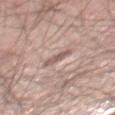The lesion was photographed on a routine skin check and not biopsied; there is no pathology result. A male patient in their mid- to late 40s. This image is a 15 mm lesion crop taken from a total-body photograph. The lesion is located on the leg.